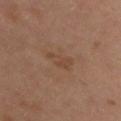{
  "biopsy_status": "not biopsied; imaged during a skin examination",
  "lighting": "cross-polarized",
  "site": "upper back",
  "patient": {
    "sex": "male",
    "age_approx": 35
  },
  "image": {
    "source": "total-body photography crop",
    "field_of_view_mm": 15
  }
}